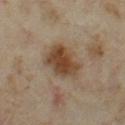This lesion was catalogued during total-body skin photography and was not selected for biopsy. The lesion is located on the left thigh. A 15 mm close-up extracted from a 3D total-body photography capture. Approximately 5 mm at its widest. The subject is a female aged 33–37.